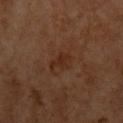No biopsy was performed on this lesion — it was imaged during a full skin examination and was not determined to be concerning. The subject is a male about 60 years old. About 3.5 mm across. This is a cross-polarized tile. A region of skin cropped from a whole-body photographic capture, roughly 15 mm wide. The lesion-visualizer software estimated border irregularity of about 3.5 on a 0–10 scale. The software also gave a nevus-likeness score of about 10/100 and lesion-presence confidence of about 100/100. On the chest.